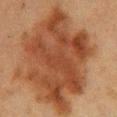Q: What is the lesion's diameter?
A: ~11.5 mm (longest diameter)
Q: What are the patient's age and sex?
A: female, about 55 years old
Q: What is the imaging modality?
A: ~15 mm tile from a whole-body skin photo
Q: What is the anatomic site?
A: the chest